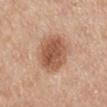This lesion was catalogued during total-body skin photography and was not selected for biopsy. The lesion is on the back. A male subject approximately 55 years of age. A lesion tile, about 15 mm wide, cut from a 3D total-body photograph.